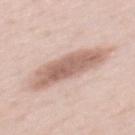Q: Is there a histopathology result?
A: no biopsy performed (imaged during a skin exam)
Q: Patient demographics?
A: female, roughly 60 years of age
Q: How was this image acquired?
A: total-body-photography crop, ~15 mm field of view
Q: What is the lesion's diameter?
A: about 8 mm
Q: Lesion location?
A: the back